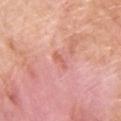The lesion was tiled from a total-body skin photograph and was not biopsied.
A roughly 15 mm field-of-view crop from a total-body skin photograph.
A male subject, roughly 60 years of age.
The lesion is located on the back.
Imaged with white-light lighting.
Longest diameter approximately 2 mm.